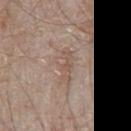No biopsy was performed on this lesion — it was imaged during a full skin examination and was not determined to be concerning. Captured under white-light illumination. A male patient aged 63–67. The lesion is on the chest. The lesion-visualizer software estimated a border-irregularity index near 5.5/10. The recorded lesion diameter is about 4.5 mm. A close-up tile cropped from a whole-body skin photograph, about 15 mm across.